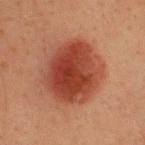Assessment:
Imaged during a routine full-body skin examination; the lesion was not biopsied and no histopathology is available.